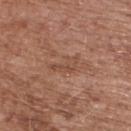Q: Was a biopsy performed?
A: total-body-photography surveillance lesion; no biopsy
Q: Lesion size?
A: ~3.5 mm (longest diameter)
Q: Lesion location?
A: the back
Q: How was this image acquired?
A: ~15 mm crop, total-body skin-cancer survey
Q: Patient demographics?
A: male, aged 73–77
Q: Illumination type?
A: white-light illumination